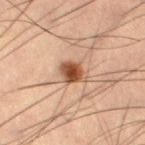biopsy status — total-body-photography surveillance lesion; no biopsy
image source — total-body-photography crop, ~15 mm field of view
tile lighting — cross-polarized illumination
subject — male, in their mid- to late 50s
anatomic site — the right thigh
diameter — ~3.5 mm (longest diameter)
image-analysis metrics — a footprint of about 6.5 mm², a shape eccentricity near 0.7, and a symmetry-axis asymmetry near 0.25; an average lesion color of about L≈44 a*≈20 b*≈30 (CIELAB), about 14 CIELAB-L* units darker than the surrounding skin, and a normalized border contrast of about 11; a within-lesion color-variation index near 4.5/10 and a peripheral color-asymmetry measure near 1; a detector confidence of about 100 out of 100 that the crop contains a lesion